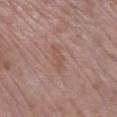Clinical summary: A 15 mm crop from a total-body photograph taken for skin-cancer surveillance. On the leg. A female patient roughly 70 years of age. Captured under white-light illumination. Approximately 3.5 mm at its widest. The total-body-photography lesion software estimated an area of roughly 4 mm² and an eccentricity of roughly 0.95. It also reported a lesion-detection confidence of about 100/100.